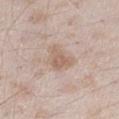The lesion was photographed on a routine skin check and not biopsied; there is no pathology result.
From the right thigh.
The patient is a male about 45 years old.
Longest diameter approximately 4 mm.
Cropped from a whole-body photographic skin survey; the tile spans about 15 mm.
This is a white-light tile.
The total-body-photography lesion software estimated an outline eccentricity of about 0.8 (0 = round, 1 = elongated) and a shape-asymmetry score of about 0.5 (0 = symmetric). And it measured a border-irregularity index near 5/10, a color-variation rating of about 2.5/10, and peripheral color asymmetry of about 1.